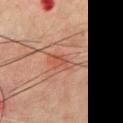The lesion was tiled from a total-body skin photograph and was not biopsied. Approximately 3 mm at its widest. This image is a 15 mm lesion crop taken from a total-body photograph. From the chest. A male subject, about 60 years old.